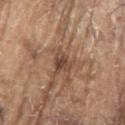Captured during whole-body skin photography for melanoma surveillance; the lesion was not biopsied. About 3.5 mm across. A male patient, in their 80s. The lesion-visualizer software estimated a footprint of about 6 mm², an eccentricity of roughly 0.4, and two-axis asymmetry of about 0.4. It also reported border irregularity of about 5 on a 0–10 scale, internal color variation of about 5 on a 0–10 scale, and peripheral color asymmetry of about 2. The software also gave a detector confidence of about 85 out of 100 that the crop contains a lesion. The lesion is located on the arm. A lesion tile, about 15 mm wide, cut from a 3D total-body photograph.